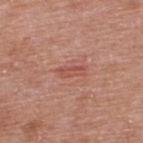Imaged during a routine full-body skin examination; the lesion was not biopsied and no histopathology is available.
A region of skin cropped from a whole-body photographic capture, roughly 15 mm wide.
The lesion is on the upper back.
Automated tile analysis of the lesion measured a lesion area of about 3.5 mm², an outline eccentricity of about 0.9 (0 = round, 1 = elongated), and a symmetry-axis asymmetry near 0.3.
A male patient about 65 years old.
Measured at roughly 3 mm in maximum diameter.